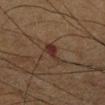biopsy_status: not biopsied; imaged during a skin examination
site: left lower leg
image:
  source: total-body photography crop
  field_of_view_mm: 15
patient:
  sex: male
  age_approx: 55
lesion_size:
  long_diameter_mm_approx: 3.0
automated_metrics:
  area_mm2_approx: 4.5
  eccentricity: 0.85
  shape_asymmetry: 0.4
  cielab_L: 27
  cielab_a: 17
  cielab_b: 21
  vs_skin_contrast_norm: 8.5
  border_irregularity_0_10: 4.0
  color_variation_0_10: 2.5
  peripheral_color_asymmetry: 1.0
  nevus_likeness_0_100: 0
  lesion_detection_confidence_0_100: 100